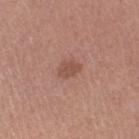The lesion was tiled from a total-body skin photograph and was not biopsied. Imaged with white-light lighting. On the left thigh. This image is a 15 mm lesion crop taken from a total-body photograph. The recorded lesion diameter is about 2.5 mm. The lesion-visualizer software estimated an area of roughly 3.5 mm², an outline eccentricity of about 0.75 (0 = round, 1 = elongated), and a shape-asymmetry score of about 0.25 (0 = symmetric). It also reported a border-irregularity index near 2.5/10, a color-variation rating of about 1/10, and radial color variation of about 0.5. It also reported a nevus-likeness score of about 40/100 and lesion-presence confidence of about 100/100. A female patient in their 50s.